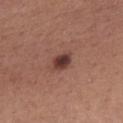Part of a total-body skin-imaging series; this lesion was reviewed on a skin check and was not flagged for biopsy.
The lesion-visualizer software estimated an area of roughly 4.5 mm² and a shape eccentricity near 0.75. And it measured an average lesion color of about L≈38 a*≈22 b*≈23 (CIELAB), roughly 13 lightness units darker than nearby skin, and a normalized lesion–skin contrast near 10.5. And it measured a classifier nevus-likeness of about 95/100 and lesion-presence confidence of about 100/100.
Longest diameter approximately 3 mm.
This is a white-light tile.
The subject is a female about 50 years old.
From the chest.
Cropped from a total-body skin-imaging series; the visible field is about 15 mm.